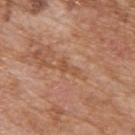Q: Is there a histopathology result?
A: catalogued during a skin exam; not biopsied
Q: Lesion location?
A: the upper back
Q: What kind of image is this?
A: ~15 mm crop, total-body skin-cancer survey
Q: Lesion size?
A: ~2.5 mm (longest diameter)
Q: What are the patient's age and sex?
A: male, aged 78 to 82